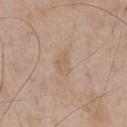Notes:
• workup · no biopsy performed (imaged during a skin exam)
• automated lesion analysis · an area of roughly 4.5 mm², an eccentricity of roughly 0.85, and a shape-asymmetry score of about 0.35 (0 = symmetric)
• location · the chest
• illumination · white-light illumination
• subject · male, aged 48 to 52
• image source · total-body-photography crop, ~15 mm field of view